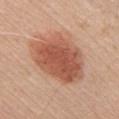workup=catalogued during a skin exam; not biopsied
tile lighting=white-light illumination
location=the chest
image=~15 mm crop, total-body skin-cancer survey
subject=male, about 40 years old
size=about 8 mm